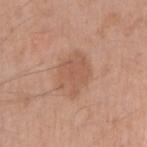Impression: Imaged during a routine full-body skin examination; the lesion was not biopsied and no histopathology is available. Image and clinical context: A close-up tile cropped from a whole-body skin photograph, about 15 mm across. The lesion-visualizer software estimated a lesion area of about 13 mm², a shape eccentricity near 0.7, and a shape-asymmetry score of about 0.2 (0 = symmetric). The analysis additionally found a lesion color around L≈56 a*≈22 b*≈30 in CIELAB. It also reported a color-variation rating of about 2/10 and radial color variation of about 0.5. The lesion is located on the right upper arm. About 5 mm across. The patient is a male about 60 years old. The tile uses white-light illumination.